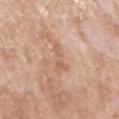<record>
<patient>
  <sex>female</sex>
  <age_approx>70</age_approx>
</patient>
<lighting>white-light</lighting>
<lesion_size>
  <long_diameter_mm_approx>4.0</long_diameter_mm_approx>
</lesion_size>
<image>
  <source>total-body photography crop</source>
  <field_of_view_mm>15</field_of_view_mm>
</image>
<site>right upper arm</site>
</record>